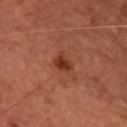Clinical summary: Captured under cross-polarized illumination. A close-up tile cropped from a whole-body skin photograph, about 15 mm across. On the upper back. A female subject aged 43–47. The lesion's longest dimension is about 2.5 mm.